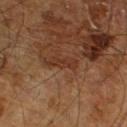Longest diameter approximately 3.5 mm. The tile uses cross-polarized illumination. Located on the leg. A male subject, aged around 65. A 15 mm close-up tile from a total-body photography series done for melanoma screening.